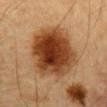notes = no biopsy performed (imaged during a skin exam) | anatomic site = the chest | subject = male, about 85 years old or older | imaging modality = total-body-photography crop, ~15 mm field of view | illumination = cross-polarized illumination | size = ≈7.5 mm.